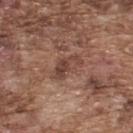  biopsy_status: not biopsied; imaged during a skin examination
  patient:
    sex: male
    age_approx: 75
  lesion_size:
    long_diameter_mm_approx: 4.0
  site: back
  image:
    source: total-body photography crop
    field_of_view_mm: 15
  automated_metrics:
    area_mm2_approx: 7.5
    eccentricity: 0.8
    shape_asymmetry: 0.3
    cielab_L: 44
    cielab_a: 20
    cielab_b: 25
    vs_skin_darker_L: 8.0
    vs_skin_contrast_norm: 6.5
    border_irregularity_0_10: 3.0
    color_variation_0_10: 6.0